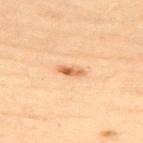No biopsy was performed on this lesion — it was imaged during a full skin examination and was not determined to be concerning. The total-body-photography lesion software estimated a footprint of about 3 mm², an eccentricity of roughly 0.95, and a shape-asymmetry score of about 0.25 (0 = symmetric). The analysis additionally found an average lesion color of about L≈66 a*≈25 b*≈42 (CIELAB), about 13 CIELAB-L* units darker than the surrounding skin, and a lesion-to-skin contrast of about 8 (normalized; higher = more distinct). And it measured a nevus-likeness score of about 100/100 and a lesion-detection confidence of about 100/100. A roughly 15 mm field-of-view crop from a total-body skin photograph. This is a cross-polarized tile. From the upper back. About 3 mm across. A female patient, about 40 years old.